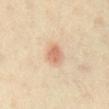{
  "biopsy_status": "not biopsied; imaged during a skin examination",
  "site": "front of the torso",
  "lighting": "cross-polarized",
  "patient": {
    "sex": "male",
    "age_approx": 65
  },
  "image": {
    "source": "total-body photography crop",
    "field_of_view_mm": 15
  },
  "automated_metrics": {
    "cielab_L": 68,
    "cielab_a": 23,
    "cielab_b": 34,
    "vs_skin_darker_L": 11.0,
    "vs_skin_contrast_norm": 7.0,
    "nevus_likeness_0_100": 95
  }
}